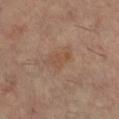Part of a total-body skin-imaging series; this lesion was reviewed on a skin check and was not flagged for biopsy. Captured under cross-polarized illumination. The lesion is located on the leg. A region of skin cropped from a whole-body photographic capture, roughly 15 mm wide. The patient is a female approximately 60 years of age. Approximately 3 mm at its widest. An algorithmic analysis of the crop reported a footprint of about 4 mm², an eccentricity of roughly 0.8, and a shape-asymmetry score of about 0.35 (0 = symmetric). And it measured an automated nevus-likeness rating near 0 out of 100 and lesion-presence confidence of about 100/100.